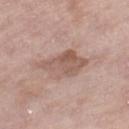Captured during whole-body skin photography for melanoma surveillance; the lesion was not biopsied. The lesion is on the right lower leg. This is a white-light tile. The recorded lesion diameter is about 5.5 mm. A 15 mm crop from a total-body photograph taken for skin-cancer surveillance. The lesion-visualizer software estimated two-axis asymmetry of about 0.3. The analysis additionally found a mean CIELAB color near L≈56 a*≈18 b*≈25, roughly 10 lightness units darker than nearby skin, and a normalized border contrast of about 6.5. It also reported an automated nevus-likeness rating near 0 out of 100 and lesion-presence confidence of about 100/100. The patient is a female in their 70s.